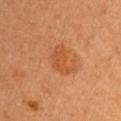{"biopsy_status": "not biopsied; imaged during a skin examination", "patient": {"sex": "female", "age_approx": 50}, "lesion_size": {"long_diameter_mm_approx": 4.0}, "lighting": "cross-polarized", "image": {"source": "total-body photography crop", "field_of_view_mm": 15}}